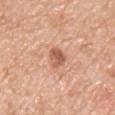workup: total-body-photography surveillance lesion; no biopsy
image source: total-body-photography crop, ~15 mm field of view
site: the chest
subject: male, aged approximately 55